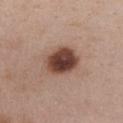workup: catalogued during a skin exam; not biopsied | body site: the chest | diameter: ~4.5 mm (longest diameter) | subject: female, aged approximately 40 | illumination: white-light | image: 15 mm crop, total-body photography | automated lesion analysis: an area of roughly 12 mm² and a shape-asymmetry score of about 0.15 (0 = symmetric); an average lesion color of about L≈43 a*≈20 b*≈25 (CIELAB), roughly 18 lightness units darker than nearby skin, and a normalized lesion–skin contrast near 13; a detector confidence of about 100 out of 100 that the crop contains a lesion.